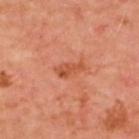Case summary:
– image-analysis metrics: a footprint of about 4 mm², an outline eccentricity of about 0.9 (0 = round, 1 = elongated), and a symmetry-axis asymmetry near 0.4; border irregularity of about 4.5 on a 0–10 scale, a color-variation rating of about 2.5/10, and radial color variation of about 0.5
– acquisition: ~15 mm crop, total-body skin-cancer survey
– site: the upper back
– subject: male, approximately 50 years of age